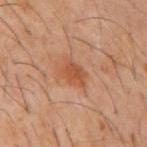This lesion was catalogued during total-body skin photography and was not selected for biopsy.
The lesion is on the mid back.
This is a cross-polarized tile.
The recorded lesion diameter is about 3.5 mm.
A male subject aged approximately 60.
This image is a 15 mm lesion crop taken from a total-body photograph.